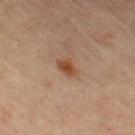Notes:
– workup — total-body-photography surveillance lesion; no biopsy
– subject — female, about 65 years old
– lesion diameter — ≈3 mm
– imaging modality — total-body-photography crop, ~15 mm field of view
– location — the leg